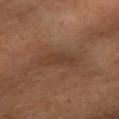– follow-up — total-body-photography surveillance lesion; no biopsy
– patient — female, aged around 45
– lighting — cross-polarized illumination
– image-analysis metrics — a lesion color around L≈38 a*≈18 b*≈27 in CIELAB, roughly 6 lightness units darker than nearby skin, and a normalized border contrast of about 6; a nevus-likeness score of about 0/100 and a detector confidence of about 85 out of 100 that the crop contains a lesion
– body site — the left arm
– image source — ~15 mm crop, total-body skin-cancer survey